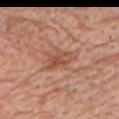Q: Is there a histopathology result?
A: total-body-photography surveillance lesion; no biopsy
Q: Patient demographics?
A: male, roughly 85 years of age or older
Q: What is the anatomic site?
A: the chest
Q: Lesion size?
A: ~3.5 mm (longest diameter)
Q: What is the imaging modality?
A: ~15 mm crop, total-body skin-cancer survey
Q: What did automated image analysis measure?
A: an area of roughly 6.5 mm², a shape eccentricity near 0.7, and a symmetry-axis asymmetry near 0.3; an average lesion color of about L≈52 a*≈25 b*≈32 (CIELAB) and about 9 CIELAB-L* units darker than the surrounding skin; border irregularity of about 4 on a 0–10 scale and radial color variation of about 1.5
Q: Illumination type?
A: white-light